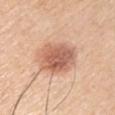imaging modality: 15 mm crop, total-body photography | site: the right upper arm | automated lesion analysis: a footprint of about 16 mm², an eccentricity of roughly 0.65, and a symmetry-axis asymmetry near 0.15; an average lesion color of about L≈61 a*≈24 b*≈31 (CIELAB), roughly 14 lightness units darker than nearby skin, and a normalized lesion–skin contrast near 8.5; an automated nevus-likeness rating near 95 out of 100 | illumination: white-light | patient: male, in their mid- to late 60s | lesion size: ~5 mm (longest diameter).